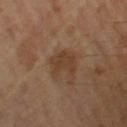Part of a total-body skin-imaging series; this lesion was reviewed on a skin check and was not flagged for biopsy.
The subject is a male in their mid- to late 60s.
On the left thigh.
A lesion tile, about 15 mm wide, cut from a 3D total-body photograph.